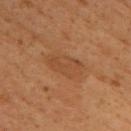* notes · no biopsy performed (imaged during a skin exam)
* location · the back
* subject · female, about 40 years old
* image · ~15 mm crop, total-body skin-cancer survey